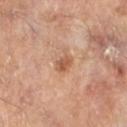Q: Was a biopsy performed?
A: catalogued during a skin exam; not biopsied
Q: Patient demographics?
A: male, aged around 65
Q: What is the anatomic site?
A: the left lower leg
Q: What is the imaging modality?
A: total-body-photography crop, ~15 mm field of view
Q: How large is the lesion?
A: about 2.5 mm
Q: How was the tile lit?
A: cross-polarized illumination
Q: What did automated image analysis measure?
A: an average lesion color of about L≈54 a*≈22 b*≈32 (CIELAB) and about 10 CIELAB-L* units darker than the surrounding skin; a border-irregularity index near 2.5/10 and a color-variation rating of about 2/10; lesion-presence confidence of about 100/100